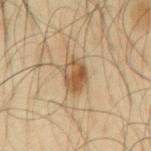Recorded during total-body skin imaging; not selected for excision or biopsy. The total-body-photography lesion software estimated a footprint of about 8 mm² and an eccentricity of roughly 0.75. It also reported an automated nevus-likeness rating near 85 out of 100 and a lesion-detection confidence of about 100/100. A male subject, in their mid- to late 60s. The lesion's longest dimension is about 4 mm. From the mid back. A 15 mm close-up tile from a total-body photography series done for melanoma screening. Imaged with cross-polarized lighting.